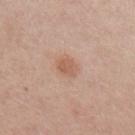Part of a total-body skin-imaging series; this lesion was reviewed on a skin check and was not flagged for biopsy.
The lesion is on the left upper arm.
The subject is a female in their 40s.
This image is a 15 mm lesion crop taken from a total-body photograph.